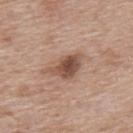Q: Is there a histopathology result?
A: total-body-photography surveillance lesion; no biopsy
Q: Where on the body is the lesion?
A: the upper back
Q: How large is the lesion?
A: ≈4.5 mm
Q: What kind of image is this?
A: 15 mm crop, total-body photography
Q: What are the patient's age and sex?
A: female, in their 40s